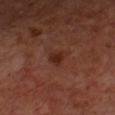follow-up = imaged on a skin check; not biopsied | image source = ~15 mm tile from a whole-body skin photo | patient = male, aged approximately 70 | tile lighting = cross-polarized | location = the chest | size = ~2.5 mm (longest diameter).